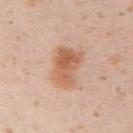workup — imaged on a skin check; not biopsied
body site — the right upper arm
tile lighting — white-light illumination
size — ~5 mm (longest diameter)
subject — female, about 20 years old
automated lesion analysis — a lesion color around L≈61 a*≈22 b*≈34 in CIELAB, about 11 CIELAB-L* units darker than the surrounding skin, and a normalized lesion–skin contrast near 8
image — ~15 mm tile from a whole-body skin photo